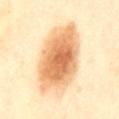Recorded during total-body skin imaging; not selected for excision or biopsy. This is a cross-polarized tile. A female patient in their mid- to late 30s. Measured at roughly 10 mm in maximum diameter. A 15 mm crop from a total-body photograph taken for skin-cancer surveillance. On the mid back.